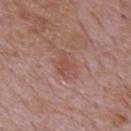Impression:
This lesion was catalogued during total-body skin photography and was not selected for biopsy.
Context:
Measured at roughly 3 mm in maximum diameter. From the mid back. A region of skin cropped from a whole-body photographic capture, roughly 15 mm wide. A male subject, about 75 years old. This is a white-light tile.